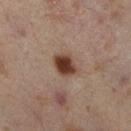Imaged during a routine full-body skin examination; the lesion was not biopsied and no histopathology is available.